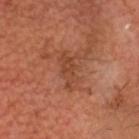Imaged during a routine full-body skin examination; the lesion was not biopsied and no histopathology is available. A male patient aged around 65. The lesion-visualizer software estimated a footprint of about 7.5 mm², an outline eccentricity of about 0.85 (0 = round, 1 = elongated), and a symmetry-axis asymmetry near 0.35. The analysis additionally found a border-irregularity rating of about 5.5/10, a within-lesion color-variation index near 2.5/10, and a peripheral color-asymmetry measure near 1. The software also gave a lesion-detection confidence of about 100/100. From the head or neck. A 15 mm crop from a total-body photograph taken for skin-cancer surveillance. The lesion's longest dimension is about 4.5 mm. This is a cross-polarized tile.